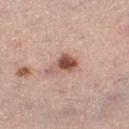follow-up: imaged on a skin check; not biopsied
patient: female, in their mid-30s
lesion size: ~3.5 mm (longest diameter)
image: total-body-photography crop, ~15 mm field of view
tile lighting: white-light
site: the left thigh